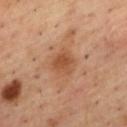The lesion was tiled from a total-body skin photograph and was not biopsied.
The total-body-photography lesion software estimated a mean CIELAB color near L≈50 a*≈23 b*≈34, a lesion–skin lightness drop of about 8, and a normalized border contrast of about 6.5. It also reported a detector confidence of about 100 out of 100 that the crop contains a lesion.
Located on the upper back.
A close-up tile cropped from a whole-body skin photograph, about 15 mm across.
A male subject in their mid- to late 50s.
The tile uses cross-polarized illumination.